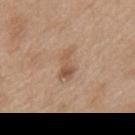{
  "biopsy_status": "not biopsied; imaged during a skin examination",
  "site": "upper back",
  "image": {
    "source": "total-body photography crop",
    "field_of_view_mm": 15
  },
  "patient": {
    "sex": "female",
    "age_approx": 40
  },
  "lesion_size": {
    "long_diameter_mm_approx": 3.5
  }
}